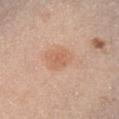Imaged during a routine full-body skin examination; the lesion was not biopsied and no histopathology is available. The tile uses white-light illumination. On the right upper arm. About 3 mm across. The patient is a female about 40 years old. A lesion tile, about 15 mm wide, cut from a 3D total-body photograph.